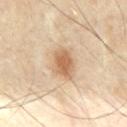Recorded during total-body skin imaging; not selected for excision or biopsy. Longest diameter approximately 3.5 mm. The lesion is on the abdomen. A 15 mm close-up extracted from a 3D total-body photography capture. The lesion-visualizer software estimated an average lesion color of about L≈61 a*≈19 b*≈34 (CIELAB) and a lesion–skin lightness drop of about 12. The analysis additionally found a classifier nevus-likeness of about 95/100. A male patient approximately 60 years of age. Imaged with cross-polarized lighting.